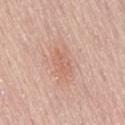The lesion was photographed on a routine skin check and not biopsied; there is no pathology result. A male patient, aged 63 to 67. A 15 mm close-up extracted from a 3D total-body photography capture. Longest diameter approximately 3.5 mm. Located on the lower back.